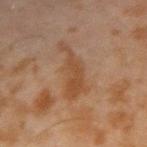Impression: Part of a total-body skin-imaging series; this lesion was reviewed on a skin check and was not flagged for biopsy. Context: An algorithmic analysis of the crop reported a lesion–skin lightness drop of about 6 and a lesion-to-skin contrast of about 6.5 (normalized; higher = more distinct). The software also gave a border-irregularity rating of about 7/10, a within-lesion color-variation index near 2/10, and radial color variation of about 0.5. The analysis additionally found a nevus-likeness score of about 0/100 and a detector confidence of about 100 out of 100 that the crop contains a lesion. On the right forearm. Approximately 6.5 mm at its widest. The patient is a male in their mid-40s. Cropped from a whole-body photographic skin survey; the tile spans about 15 mm. Captured under cross-polarized illumination.